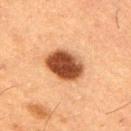follow-up: imaged on a skin check; not biopsied | subject: male, aged 48 to 52 | automated lesion analysis: an area of roughly 13 mm² and an eccentricity of roughly 0.65; a lesion color around L≈38 a*≈22 b*≈31 in CIELAB, roughly 19 lightness units darker than nearby skin, and a normalized lesion–skin contrast near 14 | location: the arm | acquisition: ~15 mm crop, total-body skin-cancer survey.